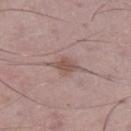| key | value |
|---|---|
| imaging modality | total-body-photography crop, ~15 mm field of view |
| lighting | white-light |
| anatomic site | the right thigh |
| automated metrics | a normalized border contrast of about 7; a border-irregularity index near 2.5/10, internal color variation of about 1 on a 0–10 scale, and peripheral color asymmetry of about 0.5; lesion-presence confidence of about 100/100 |
| subject | male, roughly 50 years of age |
| lesion size | ~3.5 mm (longest diameter) |